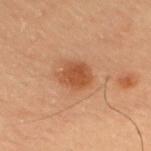No biopsy was performed on this lesion — it was imaged during a full skin examination and was not determined to be concerning. A roughly 15 mm field-of-view crop from a total-body skin photograph. The tile uses cross-polarized illumination. The patient is a male aged around 55. Automated image analysis of the tile measured an average lesion color of about L≈41 a*≈21 b*≈31 (CIELAB), a lesion–skin lightness drop of about 9, and a normalized lesion–skin contrast near 8. The software also gave internal color variation of about 2 on a 0–10 scale and a peripheral color-asymmetry measure near 0.5. The analysis additionally found an automated nevus-likeness rating near 100 out of 100. Longest diameter approximately 4 mm. The lesion is located on the upper back.